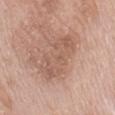workup = catalogued during a skin exam; not biopsied
tile lighting = white-light illumination
location = the mid back
size = ~6 mm (longest diameter)
image source = 15 mm crop, total-body photography
subject = female, aged 58 to 62